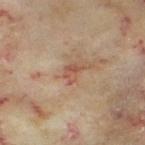- follow-up · no biopsy performed (imaged during a skin exam)
- illumination · cross-polarized
- acquisition · ~15 mm tile from a whole-body skin photo
- patient · female, aged around 60
- location · the left thigh